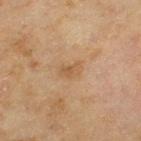<tbp_lesion>
<biopsy_status>not biopsied; imaged during a skin examination</biopsy_status>
<image>
  <source>total-body photography crop</source>
  <field_of_view_mm>15</field_of_view_mm>
</image>
<patient>
  <sex>female</sex>
  <age_approx>60</age_approx>
</patient>
<site>left thigh</site>
</tbp_lesion>